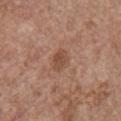<lesion>
<biopsy_status>not biopsied; imaged during a skin examination</biopsy_status>
</lesion>